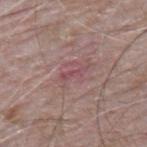* follow-up: no biopsy performed (imaged during a skin exam)
* body site: the upper back
* subject: male, aged approximately 65
* image source: ~15 mm tile from a whole-body skin photo